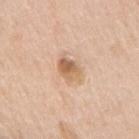Findings:
- biopsy status: total-body-photography surveillance lesion; no biopsy
- site: the mid back
- acquisition: ~15 mm tile from a whole-body skin photo
- size: ~3.5 mm (longest diameter)
- automated metrics: an area of roughly 6.5 mm², an outline eccentricity of about 0.7 (0 = round, 1 = elongated), and a symmetry-axis asymmetry near 0.25; a lesion-to-skin contrast of about 7.5 (normalized; higher = more distinct)
- patient: male, aged 63 to 67
- lighting: white-light illumination